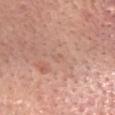{
  "biopsy_status": "not biopsied; imaged during a skin examination"
}